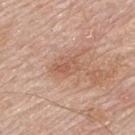{"patient": {"sex": "male", "age_approx": 70}, "image": {"source": "total-body photography crop", "field_of_view_mm": 15}, "site": "upper back"}